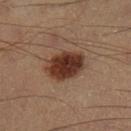biopsy status: total-body-photography surveillance lesion; no biopsy | lesion diameter: about 4.5 mm | location: the left lower leg | imaging modality: ~15 mm tile from a whole-body skin photo | tile lighting: cross-polarized illumination | patient: male, aged 38–42.